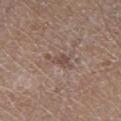Longest diameter approximately 3.5 mm. The lesion is on the right lower leg. The subject is a male aged 63–67. A region of skin cropped from a whole-body photographic capture, roughly 15 mm wide. The lesion-visualizer software estimated border irregularity of about 6.5 on a 0–10 scale and internal color variation of about 0.5 on a 0–10 scale. Captured under white-light illumination.